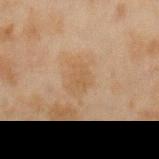The lesion was photographed on a routine skin check and not biopsied; there is no pathology result.